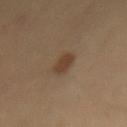Cropped from a whole-body photographic skin survey; the tile spans about 15 mm.
Located on the mid back.
A male patient approximately 40 years of age.